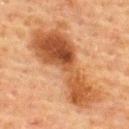biopsy_status: not biopsied; imaged during a skin examination
image:
  source: total-body photography crop
  field_of_view_mm: 15
lighting: cross-polarized
lesion_size:
  long_diameter_mm_approx: 11.0
site: upper back
patient:
  sex: female
  age_approx: 55